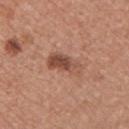Assessment: This lesion was catalogued during total-body skin photography and was not selected for biopsy. Image and clinical context: Cropped from a whole-body photographic skin survey; the tile spans about 15 mm. A female patient aged 33–37. The lesion is on the chest. The tile uses white-light illumination. An algorithmic analysis of the crop reported a lesion area of about 8.5 mm², a shape eccentricity near 0.9, and two-axis asymmetry of about 0.2. And it measured border irregularity of about 3 on a 0–10 scale, a color-variation rating of about 7/10, and a peripheral color-asymmetry measure near 2.5. And it measured a classifier nevus-likeness of about 75/100 and a lesion-detection confidence of about 100/100.